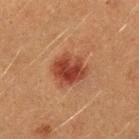Case summary:
– biopsy status — imaged on a skin check; not biopsied
– imaging modality — 15 mm crop, total-body photography
– patient — male, aged approximately 40
– lighting — cross-polarized
– site — the left forearm
– lesion size — ≈4 mm